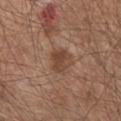Clinical impression:
No biopsy was performed on this lesion — it was imaged during a full skin examination and was not determined to be concerning.
Acquisition and patient details:
The patient is a male aged 63 to 67. Approximately 3 mm at its widest. The total-body-photography lesion software estimated a nevus-likeness score of about 50/100 and a lesion-detection confidence of about 100/100. From the chest. A close-up tile cropped from a whole-body skin photograph, about 15 mm across. Captured under white-light illumination.